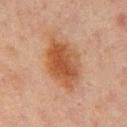The lesion was tiled from a total-body skin photograph and was not biopsied. Imaged with cross-polarized lighting. The recorded lesion diameter is about 6.5 mm. A male subject, approximately 65 years of age. A 15 mm close-up extracted from a 3D total-body photography capture. The lesion is on the mid back.